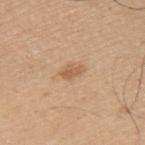This lesion was catalogued during total-body skin photography and was not selected for biopsy. From the upper back. A region of skin cropped from a whole-body photographic capture, roughly 15 mm wide. A male subject, aged approximately 45.